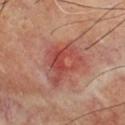  biopsy_status: not biopsied; imaged during a skin examination
  site: front of the torso
  automated_metrics:
    cielab_L: 48
    cielab_a: 28
    cielab_b: 27
    vs_skin_darker_L: 9.0
  image:
    source: total-body photography crop
    field_of_view_mm: 15
  lesion_size:
    long_diameter_mm_approx: 5.5
  patient:
    sex: male
    age_approx: 70